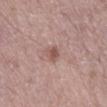No biopsy was performed on this lesion — it was imaged during a full skin examination and was not determined to be concerning. Located on the leg. The recorded lesion diameter is about 2.5 mm. The subject is a male about 70 years old. The tile uses white-light illumination. A region of skin cropped from a whole-body photographic capture, roughly 15 mm wide.